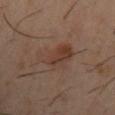Imaged during a routine full-body skin examination; the lesion was not biopsied and no histopathology is available.